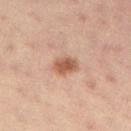| feature | finding |
|---|---|
| biopsy status | catalogued during a skin exam; not biopsied |
| TBP lesion metrics | a shape eccentricity near 0.75; about 11 CIELAB-L* units darker than the surrounding skin and a normalized lesion–skin contrast near 8.5; border irregularity of about 1.5 on a 0–10 scale, a within-lesion color-variation index near 3/10, and a peripheral color-asymmetry measure near 1; an automated nevus-likeness rating near 95 out of 100 and lesion-presence confidence of about 100/100 |
| subject | female, about 60 years old |
| site | the back |
| acquisition | ~15 mm tile from a whole-body skin photo |